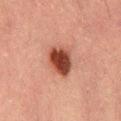{
  "patient": {
    "sex": "male",
    "age_approx": 50
  },
  "image": {
    "source": "total-body photography crop",
    "field_of_view_mm": 15
  },
  "lighting": "cross-polarized",
  "lesion_size": {
    "long_diameter_mm_approx": 4.0
  },
  "site": "lower back"
}